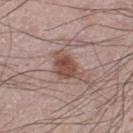{"biopsy_status": "not biopsied; imaged during a skin examination", "lesion_size": {"long_diameter_mm_approx": 4.0}, "patient": {"sex": "male", "age_approx": 60}, "image": {"source": "total-body photography crop", "field_of_view_mm": 15}, "site": "left thigh", "lighting": "white-light"}